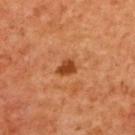Recorded during total-body skin imaging; not selected for excision or biopsy. Cropped from a total-body skin-imaging series; the visible field is about 15 mm. The patient is a female aged approximately 40. Located on the upper back.